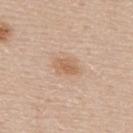| feature | finding |
|---|---|
| follow-up | catalogued during a skin exam; not biopsied |
| diameter | about 3.5 mm |
| image | total-body-photography crop, ~15 mm field of view |
| subject | male, about 60 years old |
| lighting | white-light illumination |
| automated metrics | a footprint of about 6 mm², an eccentricity of roughly 0.75, and a symmetry-axis asymmetry near 0.25; a lesion color around L≈62 a*≈18 b*≈33 in CIELAB, a lesion–skin lightness drop of about 8, and a normalized lesion–skin contrast near 6.5; a classifier nevus-likeness of about 40/100 and a lesion-detection confidence of about 100/100 |
| body site | the upper back |